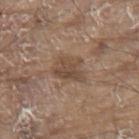biopsy status: catalogued during a skin exam; not biopsied | site: the upper back | automated metrics: a border-irregularity rating of about 4/10, a color-variation rating of about 3/10, and a peripheral color-asymmetry measure near 1; an automated nevus-likeness rating near 0 out of 100 and a lesion-detection confidence of about 100/100 | subject: male, in their 80s | image: ~15 mm tile from a whole-body skin photo.